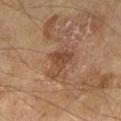<tbp_lesion>
  <biopsy_status>not biopsied; imaged during a skin examination</biopsy_status>
  <lesion_size>
    <long_diameter_mm_approx>4.5</long_diameter_mm_approx>
  </lesion_size>
  <image>
    <source>total-body photography crop</source>
    <field_of_view_mm>15</field_of_view_mm>
  </image>
  <lighting>cross-polarized</lighting>
  <patient>
    <sex>male</sex>
    <age_approx>70</age_approx>
  </patient>
  <site>left thigh</site>
</tbp_lesion>